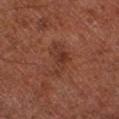follow-up=catalogued during a skin exam; not biopsied | automated metrics=a lesion color around L≈33 a*≈22 b*≈26 in CIELAB and a normalized border contrast of about 6; a border-irregularity rating of about 5.5/10, internal color variation of about 3.5 on a 0–10 scale, and peripheral color asymmetry of about 1 | anatomic site=the left lower leg | subject=male, roughly 65 years of age | imaging modality=total-body-photography crop, ~15 mm field of view | lighting=cross-polarized | lesion diameter=about 4.5 mm.